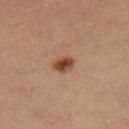A female subject in their 30s.
A region of skin cropped from a whole-body photographic capture, roughly 15 mm wide.
The tile uses cross-polarized illumination.
Automated tile analysis of the lesion measured a footprint of about 4 mm², an outline eccentricity of about 0.65 (0 = round, 1 = elongated), and a symmetry-axis asymmetry near 0.2. The analysis additionally found a border-irregularity index near 1.5/10, a color-variation rating of about 4/10, and radial color variation of about 1.5. The analysis additionally found a classifier nevus-likeness of about 100/100.
From the right thigh.